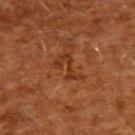Part of a total-body skin-imaging series; this lesion was reviewed on a skin check and was not flagged for biopsy. A 15 mm close-up tile from a total-body photography series done for melanoma screening. The lesion's longest dimension is about 3.5 mm. The tile uses cross-polarized illumination. On the upper back. The patient is a male about 60 years old.